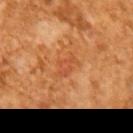No biopsy was performed on this lesion — it was imaged during a full skin examination and was not determined to be concerning.
Imaged with cross-polarized lighting.
Longest diameter approximately 3 mm.
The patient is a male in their mid- to late 60s.
Cropped from a whole-body photographic skin survey; the tile spans about 15 mm.